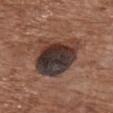The lesion was tiled from a total-body skin photograph and was not biopsied. On the chest. Imaged with white-light lighting. The lesion-visualizer software estimated a mean CIELAB color near L≈34 a*≈15 b*≈20 and a lesion–skin lightness drop of about 16. A female patient aged 73–77. This image is a 15 mm lesion crop taken from a total-body photograph. The recorded lesion diameter is about 6 mm.